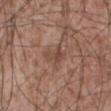Findings:
• notes · total-body-photography surveillance lesion; no biopsy
• location · the abdomen
• subject · male, aged 53–57
• diameter · about 3 mm
• illumination · white-light
• acquisition · 15 mm crop, total-body photography